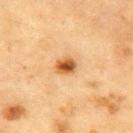biopsy status = total-body-photography surveillance lesion; no biopsy | imaging modality = ~15 mm crop, total-body skin-cancer survey | size = ≈2.5 mm | tile lighting = cross-polarized | site = the chest | patient = male, approximately 85 years of age or older.